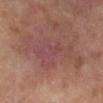Assessment:
This lesion was catalogued during total-body skin photography and was not selected for biopsy.
Image and clinical context:
The lesion's longest dimension is about 6.5 mm. This is a cross-polarized tile. A female subject, aged 58 to 62. The lesion is located on the right lower leg. A 15 mm close-up extracted from a 3D total-body photography capture.